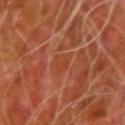notes: imaged on a skin check; not biopsied | tile lighting: cross-polarized | diameter: ≈2.5 mm | automated metrics: an area of roughly 4.5 mm², an outline eccentricity of about 0.75 (0 = round, 1 = elongated), and two-axis asymmetry of about 0.3; an average lesion color of about L≈33 a*≈24 b*≈28 (CIELAB) and roughly 4 lightness units darker than nearby skin; a classifier nevus-likeness of about 0/100 | site: the left upper arm | patient: male, aged 78 to 82 | image: 15 mm crop, total-body photography.